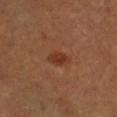The lesion was photographed on a routine skin check and not biopsied; there is no pathology result. The lesion is located on the right lower leg. A close-up tile cropped from a whole-body skin photograph, about 15 mm across. Automated tile analysis of the lesion measured a lesion area of about 4 mm², a shape eccentricity near 0.8, and a symmetry-axis asymmetry near 0.15. The analysis additionally found a mean CIELAB color near L≈35 a*≈24 b*≈31. It also reported internal color variation of about 2 on a 0–10 scale and peripheral color asymmetry of about 0.5. Captured under cross-polarized illumination. Measured at roughly 3 mm in maximum diameter. A male patient aged 48–52.